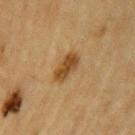notes: total-body-photography surveillance lesion; no biopsy | patient: male, about 85 years old or older | lighting: cross-polarized | location: the left upper arm | image: total-body-photography crop, ~15 mm field of view | diameter: ≈3.5 mm.